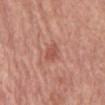Part of a total-body skin-imaging series; this lesion was reviewed on a skin check and was not flagged for biopsy. Longest diameter approximately 2.5 mm. A 15 mm close-up extracted from a 3D total-body photography capture. The subject is a male in their mid-60s. The tile uses white-light illumination. From the abdomen.